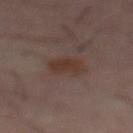notes: total-body-photography surveillance lesion; no biopsy | size: about 3.5 mm | patient: male, aged 58–62 | image-analysis metrics: a footprint of about 6.5 mm², an eccentricity of roughly 0.7, and two-axis asymmetry of about 0.25; a lesion color around L≈31 a*≈15 b*≈21 in CIELAB, roughly 6 lightness units darker than nearby skin, and a normalized lesion–skin contrast near 8 | image source: ~15 mm tile from a whole-body skin photo | site: the front of the torso.